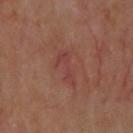Captured during whole-body skin photography for melanoma surveillance; the lesion was not biopsied.
Imaged with cross-polarized lighting.
An algorithmic analysis of the crop reported an area of roughly 5 mm², an outline eccentricity of about 0.9 (0 = round, 1 = elongated), and two-axis asymmetry of about 0.55. The software also gave a lesion color around L≈41 a*≈25 b*≈23 in CIELAB and a normalized lesion–skin contrast near 5. The software also gave border irregularity of about 7.5 on a 0–10 scale and a within-lesion color-variation index near 1/10. The software also gave an automated nevus-likeness rating near 0 out of 100.
Measured at roughly 4.5 mm in maximum diameter.
A 15 mm crop from a total-body photograph taken for skin-cancer surveillance.
The patient is a male aged 63–67.
Located on the upper back.